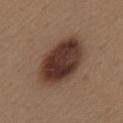- notes: total-body-photography surveillance lesion; no biopsy
- lesion size: about 7.5 mm
- illumination: white-light
- body site: the mid back
- subject: female, roughly 40 years of age
- imaging modality: ~15 mm crop, total-body skin-cancer survey